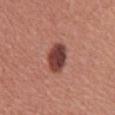Part of a total-body skin-imaging series; this lesion was reviewed on a skin check and was not flagged for biopsy. Cropped from a whole-body photographic skin survey; the tile spans about 15 mm. The patient is a male aged 43 to 47. The recorded lesion diameter is about 3.5 mm. From the abdomen. Imaged with white-light lighting. The lesion-visualizer software estimated a peripheral color-asymmetry measure near 1.5.